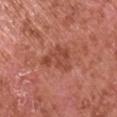Q: Is there a histopathology result?
A: total-body-photography surveillance lesion; no biopsy
Q: What kind of image is this?
A: total-body-photography crop, ~15 mm field of view
Q: What is the anatomic site?
A: the left upper arm
Q: Who is the patient?
A: male, approximately 65 years of age